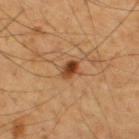<lesion>
  <biopsy_status>not biopsied; imaged during a skin examination</biopsy_status>
  <lighting>cross-polarized</lighting>
  <lesion_size>
    <long_diameter_mm_approx>2.5</long_diameter_mm_approx>
  </lesion_size>
  <patient>
    <sex>male</sex>
    <age_approx>55</age_approx>
  </patient>
  <site>upper back</site>
  <image>
    <source>total-body photography crop</source>
    <field_of_view_mm>15</field_of_view_mm>
  </image>
</lesion>